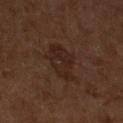  biopsy_status: not biopsied; imaged during a skin examination
  lighting: cross-polarized
  site: left lower leg
  lesion_size:
    long_diameter_mm_approx: 5.0
  image:
    source: total-body photography crop
    field_of_view_mm: 15
  patient:
    sex: male
    age_approx: 60